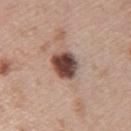Q: Is there a histopathology result?
A: no biopsy performed (imaged during a skin exam)
Q: Where on the body is the lesion?
A: the left upper arm
Q: How large is the lesion?
A: ≈3.5 mm
Q: What kind of image is this?
A: ~15 mm crop, total-body skin-cancer survey
Q: What lighting was used for the tile?
A: white-light illumination
Q: What are the patient's age and sex?
A: female, aged 48–52